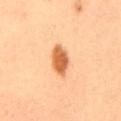size: ≈4.5 mm
image source: ~15 mm crop, total-body skin-cancer survey
subject: female, roughly 50 years of age
TBP lesion metrics: an eccentricity of roughly 0.85 and a symmetry-axis asymmetry near 0.15; a lesion color around L≈64 a*≈27 b*≈44 in CIELAB, a lesion–skin lightness drop of about 17, and a lesion-to-skin contrast of about 10 (normalized; higher = more distinct); a lesion-detection confidence of about 100/100